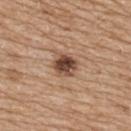Assessment: Imaged during a routine full-body skin examination; the lesion was not biopsied and no histopathology is available. Acquisition and patient details: The tile uses white-light illumination. A female subject, about 70 years old. A roughly 15 mm field-of-view crop from a total-body skin photograph. From the upper back. An algorithmic analysis of the crop reported a lesion area of about 6.5 mm², an eccentricity of roughly 0.45, and a shape-asymmetry score of about 0.2 (0 = symmetric).